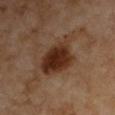{"biopsy_status": "not biopsied; imaged during a skin examination", "automated_metrics": {"cielab_L": 31, "cielab_a": 19, "cielab_b": 28, "vs_skin_darker_L": 13.0, "vs_skin_contrast_norm": 11.5, "nevus_likeness_0_100": 95, "lesion_detection_confidence_0_100": 100}, "image": {"source": "total-body photography crop", "field_of_view_mm": 15}, "patient": {"sex": "male", "age_approx": 55}, "lighting": "cross-polarized", "site": "left upper arm"}